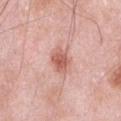anatomic site — the abdomen | image-analysis metrics — a lesion area of about 7 mm², an eccentricity of roughly 0.7, and a shape-asymmetry score of about 0.15 (0 = symmetric); about 12 CIELAB-L* units darker than the surrounding skin and a normalized border contrast of about 7.5; peripheral color asymmetry of about 1; an automated nevus-likeness rating near 85 out of 100 and a lesion-detection confidence of about 100/100 | diameter — about 3.5 mm | image — 15 mm crop, total-body photography | subject — male, aged approximately 55.